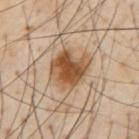<record>
  <biopsy_status>not biopsied; imaged during a skin examination</biopsy_status>
  <lighting>cross-polarized</lighting>
  <site>chest</site>
  <lesion_size>
    <long_diameter_mm_approx>5.0</long_diameter_mm_approx>
  </lesion_size>
  <image>
    <source>total-body photography crop</source>
    <field_of_view_mm>15</field_of_view_mm>
  </image>
  <patient>
    <sex>male</sex>
    <age_approx>55</age_approx>
  </patient>
</record>